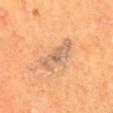{"lighting": "cross-polarized", "patient": {"sex": "male", "age_approx": 60}, "lesion_size": {"long_diameter_mm_approx": 6.0}, "site": "mid back", "image": {"source": "total-body photography crop", "field_of_view_mm": 15}}